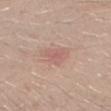  biopsy_status: not biopsied; imaged during a skin examination
  image:
    source: total-body photography crop
    field_of_view_mm: 15
  patient:
    sex: male
    age_approx: 30
  site: right forearm